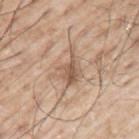Impression:
Part of a total-body skin-imaging series; this lesion was reviewed on a skin check and was not flagged for biopsy.
Background:
The patient is a male approximately 70 years of age. Located on the left upper arm. The lesion's longest dimension is about 4.5 mm. Cropped from a total-body skin-imaging series; the visible field is about 15 mm.